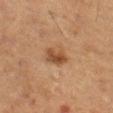Automated image analysis of the tile measured an area of roughly 5 mm², a shape eccentricity near 0.75, and a shape-asymmetry score of about 0.25 (0 = symmetric). And it measured an average lesion color of about L≈38 a*≈18 b*≈29 (CIELAB), roughly 9 lightness units darker than nearby skin, and a normalized lesion–skin contrast near 8. The analysis additionally found a border-irregularity index near 2.5/10, a color-variation rating of about 3/10, and radial color variation of about 1. It also reported an automated nevus-likeness rating near 80 out of 100 and a lesion-detection confidence of about 100/100. This is a cross-polarized tile. From the left thigh. A male subject, aged approximately 75. A roughly 15 mm field-of-view crop from a total-body skin photograph. The lesion's longest dimension is about 3 mm.